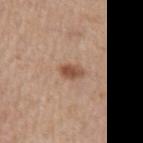– workup: catalogued during a skin exam; not biopsied
– tile lighting: white-light illumination
– body site: the left upper arm
– subject: male, aged approximately 75
– image-analysis metrics: a shape eccentricity near 0.75; a nevus-likeness score of about 95/100
– lesion diameter: about 2.5 mm
– acquisition: ~15 mm tile from a whole-body skin photo